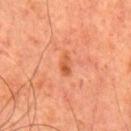workup: no biopsy performed (imaged during a skin exam) | lighting: cross-polarized | automated metrics: a lesion color around L≈51 a*≈30 b*≈38 in CIELAB, about 9 CIELAB-L* units darker than the surrounding skin, and a normalized lesion–skin contrast near 7; a within-lesion color-variation index near 2.5/10 and peripheral color asymmetry of about 1; a nevus-likeness score of about 15/100 and a detector confidence of about 100 out of 100 that the crop contains a lesion | patient: male, aged approximately 65 | image source: 15 mm crop, total-body photography | diameter: ~2.5 mm (longest diameter) | site: the front of the torso.